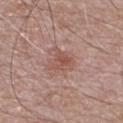{
  "biopsy_status": "not biopsied; imaged during a skin examination",
  "lighting": "white-light",
  "image": {
    "source": "total-body photography crop",
    "field_of_view_mm": 15
  },
  "patient": {
    "sex": "male",
    "age_approx": 70
  },
  "automated_metrics": {
    "nevus_likeness_0_100": 25,
    "lesion_detection_confidence_0_100": 100
  },
  "site": "chest",
  "lesion_size": {
    "long_diameter_mm_approx": 2.5
  }
}